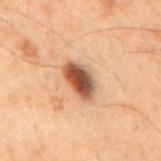Part of a total-body skin-imaging series; this lesion was reviewed on a skin check and was not flagged for biopsy.
From the mid back.
A close-up tile cropped from a whole-body skin photograph, about 15 mm across.
A male subject, in their 70s.